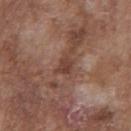The lesion was photographed on a routine skin check and not biopsied; there is no pathology result. The tile uses white-light illumination. A male patient, in their mid-70s. A 15 mm crop from a total-body photograph taken for skin-cancer surveillance. The lesion is located on the chest.